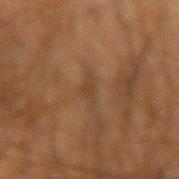notes: imaged on a skin check; not biopsied | subject: male, in their mid- to late 60s | lesion size: ≈3 mm | tile lighting: cross-polarized illumination | body site: the left forearm | image: total-body-photography crop, ~15 mm field of view | automated metrics: a lesion area of about 2 mm², an eccentricity of roughly 0.9, and a shape-asymmetry score of about 0.65 (0 = symmetric); a border-irregularity index near 6.5/10 and peripheral color asymmetry of about 0; an automated nevus-likeness rating near 0 out of 100 and a lesion-detection confidence of about 55/100.